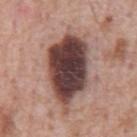Recorded during total-body skin imaging; not selected for excision or biopsy. Longest diameter approximately 9.5 mm. The lesion is located on the chest. A 15 mm close-up extracted from a 3D total-body photography capture. The patient is a male aged approximately 60. An algorithmic analysis of the crop reported a shape eccentricity near 0.85 and two-axis asymmetry of about 0.2. And it measured border irregularity of about 3 on a 0–10 scale and a peripheral color-asymmetry measure near 2. Captured under white-light illumination.